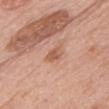Part of a total-body skin-imaging series; this lesion was reviewed on a skin check and was not flagged for biopsy. On the head or neck. The patient is a female in their 60s. About 3 mm across. A 15 mm close-up extracted from a 3D total-body photography capture. This is a white-light tile.